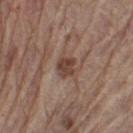- follow-up — catalogued during a skin exam; not biopsied
- imaging modality — 15 mm crop, total-body photography
- patient — male, aged 63 to 67
- location — the leg
- illumination — white-light illumination
- diameter — ~2.5 mm (longest diameter)